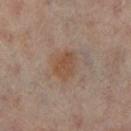biopsy status: total-body-photography surveillance lesion; no biopsy
location: the right lower leg
subject: male, aged 63 to 67
illumination: cross-polarized
TBP lesion metrics: a lesion area of about 8 mm², an outline eccentricity of about 0.65 (0 = round, 1 = elongated), and two-axis asymmetry of about 0.2; border irregularity of about 2 on a 0–10 scale, a within-lesion color-variation index near 3.5/10, and peripheral color asymmetry of about 1
image source: 15 mm crop, total-body photography